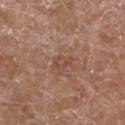Assessment:
Recorded during total-body skin imaging; not selected for excision or biopsy.
Acquisition and patient details:
On the left lower leg. Imaged with white-light lighting. Automated tile analysis of the lesion measured a border-irregularity rating of about 3/10, a color-variation rating of about 2/10, and radial color variation of about 0.5. The software also gave a classifier nevus-likeness of about 0/100 and lesion-presence confidence of about 90/100. A close-up tile cropped from a whole-body skin photograph, about 15 mm across. Longest diameter approximately 3 mm. A male subject about 75 years old.